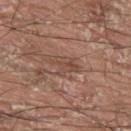Recorded during total-body skin imaging; not selected for excision or biopsy. Measured at roughly 3.5 mm in maximum diameter. From the back. A region of skin cropped from a whole-body photographic capture, roughly 15 mm wide. The patient is a male roughly 40 years of age. This is a white-light tile.